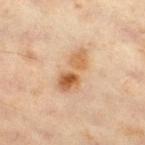workup: no biopsy performed (imaged during a skin exam)
acquisition: 15 mm crop, total-body photography
TBP lesion metrics: a lesion area of about 9 mm² and an outline eccentricity of about 0.9 (0 = round, 1 = elongated); a lesion color around L≈57 a*≈20 b*≈36 in CIELAB, a lesion–skin lightness drop of about 11, and a normalized lesion–skin contrast near 8.5; a nevus-likeness score of about 80/100 and a detector confidence of about 100 out of 100 that the crop contains a lesion
site: the right thigh
subject: male, aged 58 to 62
tile lighting: cross-polarized illumination